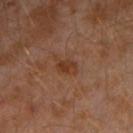workup: imaged on a skin check; not biopsied | image source: 15 mm crop, total-body photography | subject: male, about 30 years old | anatomic site: the left arm.